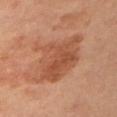Case summary:
- biopsy status — total-body-photography surveillance lesion; no biopsy
- TBP lesion metrics — a footprint of about 25 mm² and an outline eccentricity of about 0.7 (0 = round, 1 = elongated); a mean CIELAB color near L≈49 a*≈24 b*≈32, roughly 9 lightness units darker than nearby skin, and a lesion-to-skin contrast of about 6.5 (normalized; higher = more distinct); a border-irregularity rating of about 4.5/10 and a within-lesion color-variation index near 4/10
- diameter — ~7 mm (longest diameter)
- location — the left upper arm
- subject — male, in their mid- to late 60s
- lighting — cross-polarized
- image source — total-body-photography crop, ~15 mm field of view